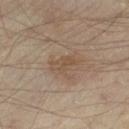Clinical impression: This lesion was catalogued during total-body skin photography and was not selected for biopsy. Context: A region of skin cropped from a whole-body photographic capture, roughly 15 mm wide. The subject is roughly 55 years of age. This is a cross-polarized tile. An algorithmic analysis of the crop reported a mean CIELAB color near L≈49 a*≈14 b*≈27, roughly 6 lightness units darker than nearby skin, and a normalized border contrast of about 5.5. It also reported a border-irregularity index near 3/10. The lesion is located on the left thigh. Longest diameter approximately 3.5 mm.